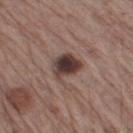Clinical impression:
The lesion was tiled from a total-body skin photograph and was not biopsied.
Image and clinical context:
A male subject, approximately 65 years of age. A lesion tile, about 15 mm wide, cut from a 3D total-body photograph. On the leg. The total-body-photography lesion software estimated an area of roughly 8.5 mm² and a shape-asymmetry score of about 0.3 (0 = symmetric). The software also gave a mean CIELAB color near L≈38 a*≈16 b*≈19 and a lesion–skin lightness drop of about 15. The software also gave a border-irregularity rating of about 3/10, internal color variation of about 7 on a 0–10 scale, and a peripheral color-asymmetry measure near 2. The analysis additionally found a nevus-likeness score of about 65/100 and a lesion-detection confidence of about 100/100. Approximately 3.5 mm at its widest. This is a white-light tile.